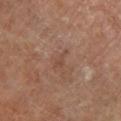Findings:
* workup — imaged on a skin check; not biopsied
* acquisition — 15 mm crop, total-body photography
* image-analysis metrics — an area of roughly 2.5 mm² and a symmetry-axis asymmetry near 0.35; a border-irregularity index near 4/10 and internal color variation of about 0 on a 0–10 scale; a detector confidence of about 100 out of 100 that the crop contains a lesion
* anatomic site — the left lower leg
* subject — female, aged approximately 65